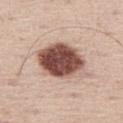workup — imaged on a skin check; not biopsied
body site — the left thigh
size — about 6 mm
patient — male, aged 63 to 67
tile lighting — white-light illumination
acquisition — total-body-photography crop, ~15 mm field of view